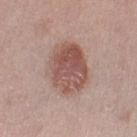follow-up: total-body-photography surveillance lesion; no biopsy
illumination: white-light illumination
anatomic site: the left lower leg
subject: male, aged around 70
acquisition: ~15 mm crop, total-body skin-cancer survey
diameter: ≈6 mm
automated lesion analysis: a footprint of about 20 mm², an eccentricity of roughly 0.65, and a shape-asymmetry score of about 0.1 (0 = symmetric); an average lesion color of about L≈53 a*≈21 b*≈24 (CIELAB), roughly 12 lightness units darker than nearby skin, and a normalized lesion–skin contrast near 8.5; a border-irregularity rating of about 1.5/10; a nevus-likeness score of about 95/100 and a lesion-detection confidence of about 100/100